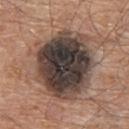workup: catalogued during a skin exam; not biopsied
diameter: about 8 mm
location: the upper back
image: total-body-photography crop, ~15 mm field of view
illumination: white-light illumination
automated lesion analysis: a lesion area of about 46 mm², an outline eccentricity of about 0.4 (0 = round, 1 = elongated), and a shape-asymmetry score of about 0.1 (0 = symmetric); a mean CIELAB color near L≈39 a*≈12 b*≈19, about 19 CIELAB-L* units darker than the surrounding skin, and a lesion-to-skin contrast of about 15.5 (normalized; higher = more distinct); a lesion-detection confidence of about 100/100
subject: male, aged around 80